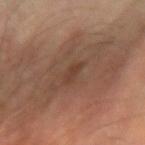Part of a total-body skin-imaging series; this lesion was reviewed on a skin check and was not flagged for biopsy.
This image is a 15 mm lesion crop taken from a total-body photograph.
About 2.5 mm across.
The patient is a male approximately 70 years of age.
From the left forearm.
Captured under cross-polarized illumination.
An algorithmic analysis of the crop reported an area of roughly 3 mm², an eccentricity of roughly 0.85, and two-axis asymmetry of about 0.4. And it measured a nevus-likeness score of about 0/100 and a detector confidence of about 100 out of 100 that the crop contains a lesion.